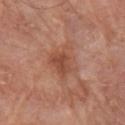<lesion>
<biopsy_status>not biopsied; imaged during a skin examination</biopsy_status>
<patient>
  <sex>male</sex>
  <age_approx>80</age_approx>
</patient>
<automated_metrics>
  <area_mm2_approx>9.5</area_mm2_approx>
  <eccentricity>0.7</eccentricity>
  <shape_asymmetry>0.35</shape_asymmetry>
  <cielab_L>49</cielab_L>
  <cielab_a>24</cielab_a>
  <cielab_b>31</cielab_b>
  <vs_skin_darker_L>9.0</vs_skin_darker_L>
  <color_variation_0_10>3.0</color_variation_0_10>
  <nevus_likeness_0_100>0</nevus_likeness_0_100>
  <lesion_detection_confidence_0_100>100</lesion_detection_confidence_0_100>
</automated_metrics>
<image>
  <source>total-body photography crop</source>
  <field_of_view_mm>15</field_of_view_mm>
</image>
<site>right upper arm</site>
<lighting>white-light</lighting>
<lesion_size>
  <long_diameter_mm_approx>4.5</long_diameter_mm_approx>
</lesion_size>
</lesion>